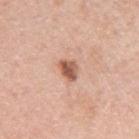Assessment:
No biopsy was performed on this lesion — it was imaged during a full skin examination and was not determined to be concerning.
Image and clinical context:
An algorithmic analysis of the crop reported a mean CIELAB color near L≈57 a*≈23 b*≈30, a lesion–skin lightness drop of about 15, and a lesion-to-skin contrast of about 9.5 (normalized; higher = more distinct). It also reported a nevus-likeness score of about 90/100. The lesion is located on the left upper arm. A close-up tile cropped from a whole-body skin photograph, about 15 mm across. Imaged with white-light lighting. A male subject, about 60 years old.